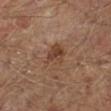biopsy status = no biopsy performed (imaged during a skin exam)
anatomic site = the left lower leg
image = total-body-photography crop, ~15 mm field of view
patient = male, approximately 65 years of age
diameter = ~3.5 mm (longest diameter)
lighting = cross-polarized illumination